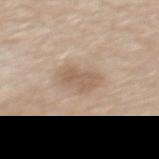biopsy status — no biopsy performed (imaged during a skin exam)
imaging modality — total-body-photography crop, ~15 mm field of view
location — the mid back
lesion diameter — ≈4 mm
patient — male, about 70 years old
illumination — white-light illumination
image-analysis metrics — a shape eccentricity near 0.85 and a symmetry-axis asymmetry near 0.2; a mean CIELAB color near L≈59 a*≈15 b*≈29, a lesion–skin lightness drop of about 8, and a normalized border contrast of about 6; a lesion-detection confidence of about 100/100